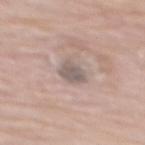biopsy status: total-body-photography surveillance lesion; no biopsy
patient: female, aged 63–67
automated lesion analysis: a lesion area of about 5.5 mm² and an eccentricity of roughly 0.5; a lesion color around L≈57 a*≈11 b*≈19 in CIELAB, roughly 10 lightness units darker than nearby skin, and a normalized lesion–skin contrast near 7
illumination: white-light illumination
location: the mid back
acquisition: ~15 mm tile from a whole-body skin photo
lesion size: about 3 mm